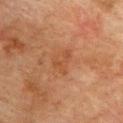Captured during whole-body skin photography for melanoma surveillance; the lesion was not biopsied. Automated image analysis of the tile measured a footprint of about 5 mm² and an outline eccentricity of about 0.65 (0 = round, 1 = elongated). About 3 mm across. A male subject in their mid- to late 70s. The tile uses cross-polarized illumination. The lesion is on the chest. A roughly 15 mm field-of-view crop from a total-body skin photograph.